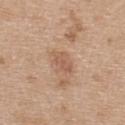<case>
<biopsy_status>not biopsied; imaged during a skin examination</biopsy_status>
<lighting>white-light</lighting>
<site>upper back</site>
<image>
  <source>total-body photography crop</source>
  <field_of_view_mm>15</field_of_view_mm>
</image>
<patient>
  <sex>female</sex>
  <age_approx>45</age_approx>
</patient>
<automated_metrics>
  <vs_skin_darker_L>8.0</vs_skin_darker_L>
  <border_irregularity_0_10>3.0</border_irregularity_0_10>
  <color_variation_0_10>2.5</color_variation_0_10>
  <peripheral_color_asymmetry>1.0</peripheral_color_asymmetry>
</automated_metrics>
<lesion_size>
  <long_diameter_mm_approx>3.0</long_diameter_mm_approx>
</lesion_size>
</case>